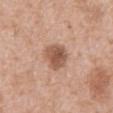{
  "biopsy_status": "not biopsied; imaged during a skin examination",
  "image": {
    "source": "total-body photography crop",
    "field_of_view_mm": 15
  },
  "site": "mid back",
  "patient": {
    "sex": "male",
    "age_approx": 60
  }
}